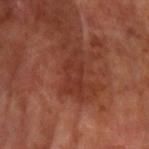Part of a total-body skin-imaging series; this lesion was reviewed on a skin check and was not flagged for biopsy. A male subject aged 63–67. The lesion is on the left upper arm. The tile uses cross-polarized illumination. Cropped from a whole-body photographic skin survey; the tile spans about 15 mm. The total-body-photography lesion software estimated a footprint of about 9 mm², an outline eccentricity of about 0.9 (0 = round, 1 = elongated), and two-axis asymmetry of about 0.35. The analysis additionally found a border-irregularity index near 5/10, a within-lesion color-variation index near 2/10, and peripheral color asymmetry of about 0.5. The analysis additionally found an automated nevus-likeness rating near 0 out of 100 and a detector confidence of about 100 out of 100 that the crop contains a lesion.